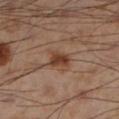No biopsy was performed on this lesion — it was imaged during a full skin examination and was not determined to be concerning.